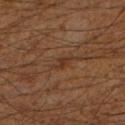  biopsy_status: not biopsied; imaged during a skin examination
  image:
    source: total-body photography crop
    field_of_view_mm: 15
  lighting: cross-polarized
  lesion_size:
    long_diameter_mm_approx: 3.0
  site: left lower leg
  patient:
    sex: male
    age_approx: 60